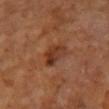Assessment: Part of a total-body skin-imaging series; this lesion was reviewed on a skin check and was not flagged for biopsy. Image and clinical context: A female patient aged approximately 70. A 15 mm close-up extracted from a 3D total-body photography capture. The tile uses cross-polarized illumination. Located on the arm.